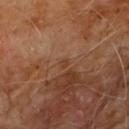| feature | finding |
|---|---|
| workup | no biopsy performed (imaged during a skin exam) |
| anatomic site | the chest |
| subject | male, aged approximately 60 |
| acquisition | ~15 mm tile from a whole-body skin photo |
| lighting | cross-polarized |